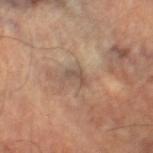Case summary:
• notes · imaged on a skin check; not biopsied
• lighting · cross-polarized
• location · the leg
• patient · male, roughly 60 years of age
• image source · total-body-photography crop, ~15 mm field of view